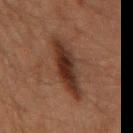Clinical impression:
Captured during whole-body skin photography for melanoma surveillance; the lesion was not biopsied.
Acquisition and patient details:
A male patient, aged 58–62. This is a cross-polarized tile. Approximately 7 mm at its widest. From the mid back. Cropped from a whole-body photographic skin survey; the tile spans about 15 mm.